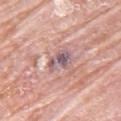Findings:
- notes · no biopsy performed (imaged during a skin exam)
- image · 15 mm crop, total-body photography
- anatomic site · the back
- TBP lesion metrics · an average lesion color of about L≈57 a*≈19 b*≈16 (CIELAB) and about 11 CIELAB-L* units darker than the surrounding skin; border irregularity of about 3.5 on a 0–10 scale, a within-lesion color-variation index near 5/10, and peripheral color asymmetry of about 1.5
- patient · female, approximately 65 years of age
- illumination · white-light
- diameter · ~3.5 mm (longest diameter)